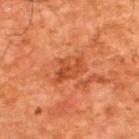biopsy status: total-body-photography surveillance lesion; no biopsy | patient: male, aged 58–62 | tile lighting: cross-polarized | TBP lesion metrics: a border-irregularity rating of about 3.5/10, internal color variation of about 4 on a 0–10 scale, and peripheral color asymmetry of about 1.5 | lesion diameter: ~4 mm (longest diameter) | image: 15 mm crop, total-body photography | anatomic site: the back.